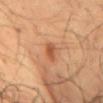  biopsy_status: not biopsied; imaged during a skin examination
  patient:
    sex: male
    age_approx: 50
  site: abdomen
  image:
    source: total-body photography crop
    field_of_view_mm: 15